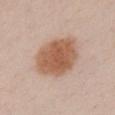follow-up: catalogued during a skin exam; not biopsied | tile lighting: white-light illumination | anatomic site: the chest | image: total-body-photography crop, ~15 mm field of view | subject: female, aged 23–27 | size: about 6.5 mm | image-analysis metrics: about 13 CIELAB-L* units darker than the surrounding skin and a lesion-to-skin contrast of about 9.5 (normalized; higher = more distinct); a detector confidence of about 100 out of 100 that the crop contains a lesion.